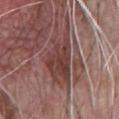notes — total-body-photography surveillance lesion; no biopsy | image-analysis metrics — a footprint of about 17 mm² and a symmetry-axis asymmetry near 0.35; an average lesion color of about L≈41 a*≈22 b*≈21 (CIELAB), roughly 11 lightness units darker than nearby skin, and a lesion-to-skin contrast of about 9 (normalized; higher = more distinct); an automated nevus-likeness rating near 0 out of 100 and lesion-presence confidence of about 85/100 | site — the front of the torso | image source — ~15 mm tile from a whole-body skin photo | lighting — white-light | patient — male, approximately 70 years of age.